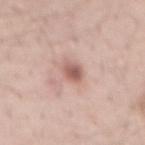follow-up — no biopsy performed (imaged during a skin exam); lesion diameter — about 2.5 mm; subject — male, aged 38 to 42; imaging modality — 15 mm crop, total-body photography; automated lesion analysis — a lesion-detection confidence of about 100/100; location — the mid back.